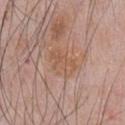Impression:
This lesion was catalogued during total-body skin photography and was not selected for biopsy.
Context:
From the front of the torso. A male subject aged approximately 60. A 15 mm close-up tile from a total-body photography series done for melanoma screening. This is a white-light tile.